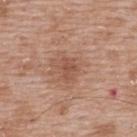Automated image analysis of the tile measured a lesion area of about 4 mm², an outline eccentricity of about 0.65 (0 = round, 1 = elongated), and two-axis asymmetry of about 0.3. The analysis additionally found a lesion–skin lightness drop of about 8 and a normalized lesion–skin contrast near 6. The software also gave a color-variation rating of about 1.5/10 and peripheral color asymmetry of about 0.5. A male patient, aged around 50. Cropped from a total-body skin-imaging series; the visible field is about 15 mm. Longest diameter approximately 2.5 mm. The lesion is on the upper back. This is a white-light tile.